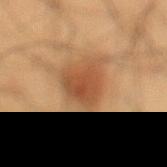Findings:
- notes — total-body-photography surveillance lesion; no biopsy
- image-analysis metrics — a mean CIELAB color near L≈46 a*≈21 b*≈33, a lesion–skin lightness drop of about 10, and a normalized border contrast of about 7.5; a border-irregularity rating of about 3/10, a color-variation rating of about 4/10, and peripheral color asymmetry of about 1; a nevus-likeness score of about 90/100 and a detector confidence of about 100 out of 100 that the crop contains a lesion
- acquisition — total-body-photography crop, ~15 mm field of view
- body site — the left lower leg
- patient — male, aged approximately 35
- lighting — cross-polarized
- diameter — ≈5 mm